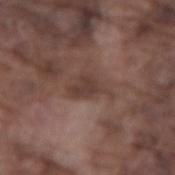Q: Is there a histopathology result?
A: total-body-photography surveillance lesion; no biopsy
Q: What kind of image is this?
A: total-body-photography crop, ~15 mm field of view
Q: Lesion size?
A: about 4.5 mm
Q: Where on the body is the lesion?
A: the right forearm
Q: What are the patient's age and sex?
A: male, aged 73 to 77